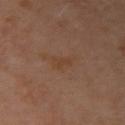{"patient": {"sex": "female", "age_approx": 50}, "lesion_size": {"long_diameter_mm_approx": 3.0}, "site": "left upper arm", "image": {"source": "total-body photography crop", "field_of_view_mm": 15}, "automated_metrics": {"area_mm2_approx": 3.0, "eccentricity": 0.9, "shape_asymmetry": 0.35}, "lighting": "cross-polarized"}